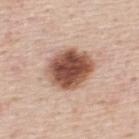biopsy status — catalogued during a skin exam; not biopsied | patient — male, aged approximately 45 | lesion size — about 5.5 mm | automated lesion analysis — an outline eccentricity of about 0.55 (0 = round, 1 = elongated) and a symmetry-axis asymmetry near 0.1; a mean CIELAB color near L≈52 a*≈22 b*≈28, roughly 20 lightness units darker than nearby skin, and a lesion-to-skin contrast of about 12.5 (normalized; higher = more distinct); a color-variation rating of about 6.5/10 and peripheral color asymmetry of about 2; a nevus-likeness score of about 100/100 | image — total-body-photography crop, ~15 mm field of view | body site — the back | illumination — white-light.